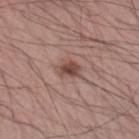This lesion was catalogued during total-body skin photography and was not selected for biopsy.
An algorithmic analysis of the crop reported an area of roughly 4.5 mm², an outline eccentricity of about 0.65 (0 = round, 1 = elongated), and a shape-asymmetry score of about 0.25 (0 = symmetric). The software also gave a mean CIELAB color near L≈47 a*≈20 b*≈23, roughly 11 lightness units darker than nearby skin, and a lesion-to-skin contrast of about 8 (normalized; higher = more distinct).
Imaged with white-light lighting.
A male subject approximately 70 years of age.
On the left upper arm.
Cropped from a total-body skin-imaging series; the visible field is about 15 mm.
Approximately 2.5 mm at its widest.